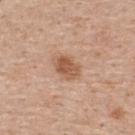notes: imaged on a skin check; not biopsied | patient: male, about 55 years old | anatomic site: the upper back | lighting: white-light | image-analysis metrics: a footprint of about 6.5 mm², a shape eccentricity near 0.75, and a symmetry-axis asymmetry near 0.25; an average lesion color of about L≈56 a*≈21 b*≈33 (CIELAB), about 11 CIELAB-L* units darker than the surrounding skin, and a normalized border contrast of about 8; a color-variation rating of about 3.5/10 | lesion size: ~3.5 mm (longest diameter) | image: total-body-photography crop, ~15 mm field of view.